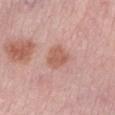| key | value |
|---|---|
| workup | catalogued during a skin exam; not biopsied |
| diameter | about 3 mm |
| acquisition | total-body-photography crop, ~15 mm field of view |
| anatomic site | the left lower leg |
| illumination | white-light |
| subject | female, roughly 45 years of age |